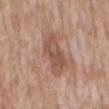Q: Was a biopsy performed?
A: imaged on a skin check; not biopsied
Q: What did automated image analysis measure?
A: a lesion color around L≈52 a*≈20 b*≈27 in CIELAB and a lesion–skin lightness drop of about 10; border irregularity of about 3.5 on a 0–10 scale, a color-variation rating of about 3.5/10, and a peripheral color-asymmetry measure near 1.5
Q: What are the patient's age and sex?
A: male, aged 58 to 62
Q: What is the lesion's diameter?
A: ~5.5 mm (longest diameter)
Q: How was this image acquired?
A: ~15 mm crop, total-body skin-cancer survey
Q: What is the anatomic site?
A: the abdomen
Q: How was the tile lit?
A: white-light illumination